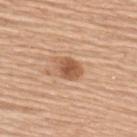Q: Is there a histopathology result?
A: catalogued during a skin exam; not biopsied
Q: What are the patient's age and sex?
A: female, about 60 years old
Q: Lesion location?
A: the upper back
Q: What lighting was used for the tile?
A: white-light illumination
Q: How large is the lesion?
A: about 3.5 mm
Q: Automated lesion metrics?
A: a footprint of about 6.5 mm², a shape eccentricity near 0.7, and two-axis asymmetry of about 0.25; border irregularity of about 2.5 on a 0–10 scale, a color-variation rating of about 3.5/10, and a peripheral color-asymmetry measure near 1
Q: What is the imaging modality?
A: total-body-photography crop, ~15 mm field of view